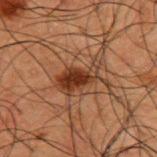Acquisition and patient details:
An algorithmic analysis of the crop reported a border-irregularity index near 5/10, a within-lesion color-variation index near 5.5/10, and a peripheral color-asymmetry measure near 1.5. The analysis additionally found an automated nevus-likeness rating near 100 out of 100 and lesion-presence confidence of about 100/100. A 15 mm close-up tile from a total-body photography series done for melanoma screening. Located on the upper back. The subject is a male about 50 years old. The tile uses cross-polarized illumination. The lesion's longest dimension is about 5.5 mm.